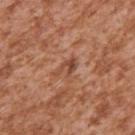Q: What did automated image analysis measure?
A: about 9 CIELAB-L* units darker than the surrounding skin; border irregularity of about 6.5 on a 0–10 scale and peripheral color asymmetry of about 0
Q: Who is the patient?
A: male, roughly 45 years of age
Q: Lesion location?
A: the right upper arm
Q: Illumination type?
A: white-light
Q: How was this image acquired?
A: 15 mm crop, total-body photography
Q: Lesion size?
A: ≈3 mm